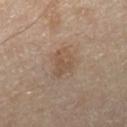No biopsy was performed on this lesion — it was imaged during a full skin examination and was not determined to be concerning.
A lesion tile, about 15 mm wide, cut from a 3D total-body photograph.
The lesion is on the right lower leg.
A male subject, in their mid-50s.